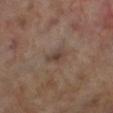Captured during whole-body skin photography for melanoma surveillance; the lesion was not biopsied. A male patient aged around 70. This is a cross-polarized tile. From the left lower leg. Cropped from a total-body skin-imaging series; the visible field is about 15 mm. Approximately 2.5 mm at its widest. Automated image analysis of the tile measured a lesion area of about 3.5 mm², an eccentricity of roughly 0.8, and a shape-asymmetry score of about 0.4 (0 = symmetric). The software also gave border irregularity of about 3.5 on a 0–10 scale, internal color variation of about 2 on a 0–10 scale, and peripheral color asymmetry of about 0.5. The software also gave a classifier nevus-likeness of about 0/100 and lesion-presence confidence of about 100/100.